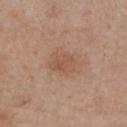The lesion was photographed on a routine skin check and not biopsied; there is no pathology result. The lesion is on the chest. The patient is a female roughly 55 years of age. This image is a 15 mm lesion crop taken from a total-body photograph.